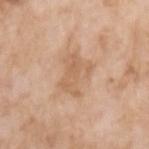notes: catalogued during a skin exam; not biopsied | imaging modality: 15 mm crop, total-body photography | location: the left upper arm | automated lesion analysis: an outline eccentricity of about 0.75 (0 = round, 1 = elongated) and two-axis asymmetry of about 0.45; border irregularity of about 6.5 on a 0–10 scale, internal color variation of about 2.5 on a 0–10 scale, and peripheral color asymmetry of about 1; lesion-presence confidence of about 100/100 | tile lighting: white-light | lesion diameter: ≈4.5 mm | patient: female, in their mid-70s.